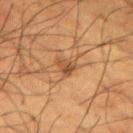The lesion was photographed on a routine skin check and not biopsied; there is no pathology result.
Imaged with cross-polarized lighting.
A male patient aged around 60.
About 2.5 mm across.
A region of skin cropped from a whole-body photographic capture, roughly 15 mm wide.
Located on the left upper arm.